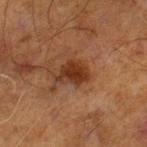  biopsy_status: not biopsied; imaged during a skin examination
  lesion_size:
    long_diameter_mm_approx: 3.5
  patient:
    sex: male
    age_approx: 70
  site: leg
  image:
    source: total-body photography crop
    field_of_view_mm: 15
  lighting: cross-polarized